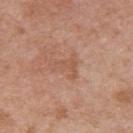Clinical impression: This lesion was catalogued during total-body skin photography and was not selected for biopsy. Image and clinical context: From the upper back. Cropped from a whole-body photographic skin survey; the tile spans about 15 mm. Imaged with white-light lighting. Measured at roughly 3.5 mm in maximum diameter. The patient is a female in their 60s.